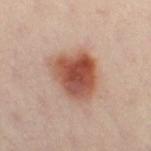The lesion was photographed on a routine skin check and not biopsied; there is no pathology result.
A roughly 15 mm field-of-view crop from a total-body skin photograph.
From the left leg.
About 5.5 mm across.
A female patient, aged 38 to 42.